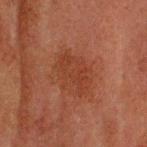biopsy status: catalogued during a skin exam; not biopsied | image-analysis metrics: a lesion area of about 14 mm², an outline eccentricity of about 0.7 (0 = round, 1 = elongated), and a symmetry-axis asymmetry near 0.2; border irregularity of about 2.5 on a 0–10 scale and a within-lesion color-variation index near 2.5/10; an automated nevus-likeness rating near 0 out of 100 and a lesion-detection confidence of about 100/100 | anatomic site: the head or neck | patient: male, aged 58–62 | diameter: ~5 mm (longest diameter) | image: ~15 mm tile from a whole-body skin photo.